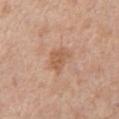{
  "biopsy_status": "not biopsied; imaged during a skin examination",
  "patient": {
    "sex": "male",
    "age_approx": 55
  },
  "automated_metrics": {
    "cielab_L": 58,
    "cielab_a": 21,
    "cielab_b": 33,
    "vs_skin_contrast_norm": 6.0
  },
  "lighting": "white-light",
  "lesion_size": {
    "long_diameter_mm_approx": 3.5
  },
  "site": "left upper arm",
  "image": {
    "source": "total-body photography crop",
    "field_of_view_mm": 15
  }
}